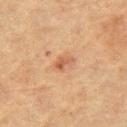Notes:
• workup · imaged on a skin check; not biopsied
• body site · the left thigh
• lighting · cross-polarized
• patient · female, aged 53 to 57
• automated lesion analysis · a lesion area of about 3.5 mm² and two-axis asymmetry of about 0.3
• image · total-body-photography crop, ~15 mm field of view